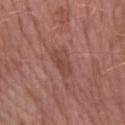follow-up — no biopsy performed (imaged during a skin exam)
location — the left forearm
image source — ~15 mm tile from a whole-body skin photo
lighting — white-light
image-analysis metrics — a lesion area of about 6 mm², an outline eccentricity of about 0.85 (0 = round, 1 = elongated), and a symmetry-axis asymmetry near 0.3; an average lesion color of about L≈45 a*≈24 b*≈26 (CIELAB), about 7 CIELAB-L* units darker than the surrounding skin, and a normalized border contrast of about 6
subject — female, about 60 years old